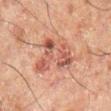| field | value |
|---|---|
| follow-up | total-body-photography surveillance lesion; no biopsy |
| image source | ~15 mm tile from a whole-body skin photo |
| TBP lesion metrics | an area of roughly 18 mm² and a shape-asymmetry score of about 0.45 (0 = symmetric); a mean CIELAB color near L≈43 a*≈20 b*≈24 and a normalized border contrast of about 6.5; a border-irregularity rating of about 5.5/10, internal color variation of about 6.5 on a 0–10 scale, and peripheral color asymmetry of about 2 |
| patient | male, aged approximately 60 |
| body site | the left leg |
| lesion diameter | ≈6.5 mm |
| lighting | cross-polarized illumination |